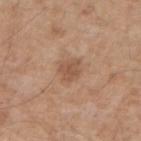Assessment: The lesion was tiled from a total-body skin photograph and was not biopsied.